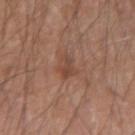biopsy_status: not biopsied; imaged during a skin examination
patient:
  sex: male
  age_approx: 65
site: left forearm
image:
  source: total-body photography crop
  field_of_view_mm: 15